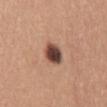Part of a total-body skin-imaging series; this lesion was reviewed on a skin check and was not flagged for biopsy.
Automated image analysis of the tile measured an average lesion color of about L≈44 a*≈21 b*≈25 (CIELAB) and roughly 20 lightness units darker than nearby skin.
A female patient aged 28–32.
A roughly 15 mm field-of-view crop from a total-body skin photograph.
On the mid back.
The recorded lesion diameter is about 4 mm.